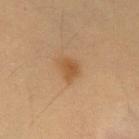Part of a total-body skin-imaging series; this lesion was reviewed on a skin check and was not flagged for biopsy. The lesion is on the abdomen. The total-body-photography lesion software estimated an area of roughly 4.5 mm², an outline eccentricity of about 0.7 (0 = round, 1 = elongated), and a shape-asymmetry score of about 0.35 (0 = symmetric). It also reported a border-irregularity rating of about 3/10 and peripheral color asymmetry of about 0.5. The lesion's longest dimension is about 3 mm. A male subject in their mid-50s. A roughly 15 mm field-of-view crop from a total-body skin photograph. The tile uses cross-polarized illumination.